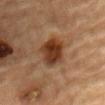The lesion was tiled from a total-body skin photograph and was not biopsied. The lesion is located on the mid back. Captured under cross-polarized illumination. The total-body-photography lesion software estimated a footprint of about 11 mm², an outline eccentricity of about 0.55 (0 = round, 1 = elongated), and two-axis asymmetry of about 0.15. The analysis additionally found a mean CIELAB color near L≈32 a*≈19 b*≈28, a lesion–skin lightness drop of about 12, and a normalized lesion–skin contrast near 11. It also reported a classifier nevus-likeness of about 95/100 and a lesion-detection confidence of about 100/100. A male patient aged around 85 or older. A region of skin cropped from a whole-body photographic capture, roughly 15 mm wide. Measured at roughly 4 mm in maximum diameter.